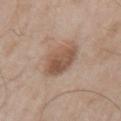biopsy status = imaged on a skin check; not biopsied
subject = male, in their mid- to late 50s
size = ≈4.5 mm
imaging modality = ~15 mm tile from a whole-body skin photo
body site = the left upper arm
illumination = white-light illumination